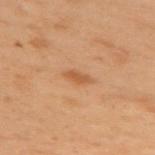Q: Was a biopsy performed?
A: imaged on a skin check; not biopsied
Q: How was this image acquired?
A: ~15 mm tile from a whole-body skin photo
Q: What is the lesion's diameter?
A: about 3 mm
Q: What are the patient's age and sex?
A: female, in their mid-50s
Q: Automated lesion metrics?
A: border irregularity of about 3 on a 0–10 scale, a color-variation rating of about 1/10, and radial color variation of about 0.5
Q: Where on the body is the lesion?
A: the left arm
Q: Illumination type?
A: cross-polarized illumination